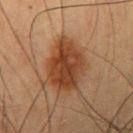Assessment:
Imaged during a routine full-body skin examination; the lesion was not biopsied and no histopathology is available.
Context:
Imaged with cross-polarized lighting. Cropped from a whole-body photographic skin survey; the tile spans about 15 mm. Approximately 6 mm at its widest. A male subject roughly 55 years of age. From the right upper arm.